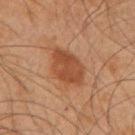Q: Was a biopsy performed?
A: no biopsy performed (imaged during a skin exam)
Q: What is the imaging modality?
A: 15 mm crop, total-body photography
Q: Illumination type?
A: cross-polarized
Q: Who is the patient?
A: male, aged 63 to 67
Q: What did automated image analysis measure?
A: a lesion–skin lightness drop of about 8 and a normalized lesion–skin contrast near 7.5; a classifier nevus-likeness of about 95/100
Q: Where on the body is the lesion?
A: the back